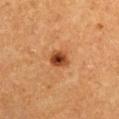Q: Was this lesion biopsied?
A: imaged on a skin check; not biopsied
Q: Illumination type?
A: cross-polarized illumination
Q: What is the imaging modality?
A: total-body-photography crop, ~15 mm field of view
Q: Patient demographics?
A: male, about 75 years old
Q: Lesion location?
A: the right upper arm
Q: Lesion size?
A: about 2.5 mm
Q: What did automated image analysis measure?
A: a lesion area of about 4.5 mm², an outline eccentricity of about 0.55 (0 = round, 1 = elongated), and two-axis asymmetry of about 0.2; an average lesion color of about L≈36 a*≈23 b*≈33 (CIELAB) and a lesion–skin lightness drop of about 13; an automated nevus-likeness rating near 100 out of 100 and lesion-presence confidence of about 100/100